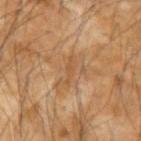Notes:
– lighting · cross-polarized
– automated metrics · a footprint of about 2.5 mm² and two-axis asymmetry of about 0.45; an average lesion color of about L≈49 a*≈20 b*≈35 (CIELAB), about 6 CIELAB-L* units darker than the surrounding skin, and a normalized border contrast of about 5; border irregularity of about 6 on a 0–10 scale and radial color variation of about 0; a classifier nevus-likeness of about 0/100 and a lesion-detection confidence of about 60/100
– lesion diameter · about 3 mm
– patient · male, in their mid-50s
– image source · ~15 mm crop, total-body skin-cancer survey
– site · the left forearm